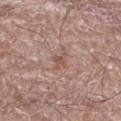Impression: Recorded during total-body skin imaging; not selected for excision or biopsy.